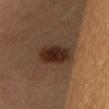Clinical impression: Captured during whole-body skin photography for melanoma surveillance; the lesion was not biopsied. Image and clinical context: The lesion is located on the head or neck. Imaged with cross-polarized lighting. Measured at roughly 4.5 mm in maximum diameter. A female subject about 35 years old. A close-up tile cropped from a whole-body skin photograph, about 15 mm across.